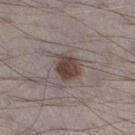{
  "biopsy_status": "not biopsied; imaged during a skin examination",
  "automated_metrics": {
    "peripheral_color_asymmetry": 1.5,
    "nevus_likeness_0_100": 95,
    "lesion_detection_confidence_0_100": 100
  },
  "lighting": "white-light",
  "image": {
    "source": "total-body photography crop",
    "field_of_view_mm": 15
  },
  "site": "left lower leg",
  "patient": {
    "sex": "male",
    "age_approx": 70
  }
}